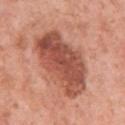{"biopsy_status": "not biopsied; imaged during a skin examination", "patient": {"sex": "female", "age_approx": 50}, "image": {"source": "total-body photography crop", "field_of_view_mm": 15}, "site": "left upper arm"}